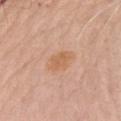{"biopsy_status": "not biopsied; imaged during a skin examination", "lesion_size": {"long_diameter_mm_approx": 2.5}, "site": "chest", "lighting": "white-light", "image": {"source": "total-body photography crop", "field_of_view_mm": 15}, "patient": {"sex": "male", "age_approx": 80}}